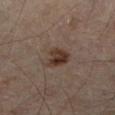Part of a total-body skin-imaging series; this lesion was reviewed on a skin check and was not flagged for biopsy. From the right lower leg. A 15 mm close-up tile from a total-body photography series done for melanoma screening. A male patient, in their mid-60s.